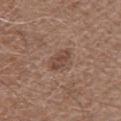body site=the mid back
lighting=white-light illumination
patient=male, aged 73–77
automated lesion analysis=a lesion area of about 4 mm² and an eccentricity of roughly 0.8; a mean CIELAB color near L≈45 a*≈19 b*≈26, roughly 9 lightness units darker than nearby skin, and a normalized lesion–skin contrast near 7; an automated nevus-likeness rating near 5 out of 100
diameter=≈3 mm
image source=~15 mm crop, total-body skin-cancer survey